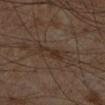* follow-up · total-body-photography surveillance lesion; no biopsy
* size · ~3.5 mm (longest diameter)
* subject · male, aged 58 to 62
* site · the leg
* tile lighting · cross-polarized
* image source · total-body-photography crop, ~15 mm field of view